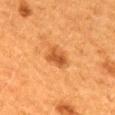| feature | finding |
|---|---|
| workup | imaged on a skin check; not biopsied |
| size | about 3 mm |
| patient | female, aged around 40 |
| acquisition | ~15 mm tile from a whole-body skin photo |
| anatomic site | the mid back |